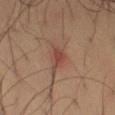Captured during whole-body skin photography for melanoma surveillance; the lesion was not biopsied.
About 4 mm across.
A region of skin cropped from a whole-body photographic capture, roughly 15 mm wide.
A male patient in their 40s.
Captured under cross-polarized illumination.
The lesion is located on the leg.
The lesion-visualizer software estimated an area of roughly 6 mm², an eccentricity of roughly 0.8, and two-axis asymmetry of about 0.35. It also reported an average lesion color of about L≈46 a*≈22 b*≈27 (CIELAB) and roughly 8 lightness units darker than nearby skin.